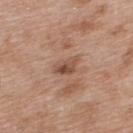Clinical impression:
The lesion was tiled from a total-body skin photograph and was not biopsied.
Background:
The subject is a female approximately 40 years of age. On the upper back. Captured under white-light illumination. Cropped from a whole-body photographic skin survey; the tile spans about 15 mm.